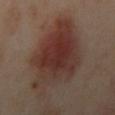Captured under cross-polarized illumination. The lesion is on the left arm. A close-up tile cropped from a whole-body skin photograph, about 15 mm across. The lesion-visualizer software estimated a lesion area of about 42 mm², an eccentricity of roughly 0.55, and a shape-asymmetry score of about 0.3 (0 = symmetric). The software also gave a lesion color around L≈34 a*≈19 b*≈23 in CIELAB, about 10 CIELAB-L* units darker than the surrounding skin, and a lesion-to-skin contrast of about 9 (normalized; higher = more distinct). And it measured border irregularity of about 4 on a 0–10 scale, a within-lesion color-variation index near 7/10, and peripheral color asymmetry of about 2.5. It also reported an automated nevus-likeness rating near 100 out of 100 and lesion-presence confidence of about 100/100. The subject is a female aged 38 to 42. About 8.5 mm across.